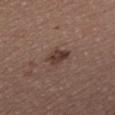Clinical impression: No biopsy was performed on this lesion — it was imaged during a full skin examination and was not determined to be concerning. Image and clinical context: The recorded lesion diameter is about 3 mm. The lesion is located on the chest. A male patient aged around 40. Captured under white-light illumination. Automated tile analysis of the lesion measured an area of roughly 5 mm², an eccentricity of roughly 0.75, and two-axis asymmetry of about 0.25. The analysis additionally found a border-irregularity rating of about 2.5/10 and a color-variation rating of about 3/10. It also reported a detector confidence of about 100 out of 100 that the crop contains a lesion. A lesion tile, about 15 mm wide, cut from a 3D total-body photograph.